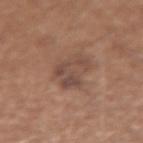The lesion was tiled from a total-body skin photograph and was not biopsied. Cropped from a total-body skin-imaging series; the visible field is about 15 mm. The patient is a female roughly 40 years of age. The lesion-visualizer software estimated a classifier nevus-likeness of about 5/100 and a lesion-detection confidence of about 100/100. On the left forearm. The lesion's longest dimension is about 5 mm. Captured under white-light illumination.